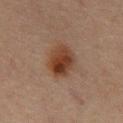• biopsy status — imaged on a skin check; not biopsied
• patient — male, about 70 years old
• tile lighting — cross-polarized illumination
• lesion size — ~4.5 mm (longest diameter)
• body site — the mid back
• acquisition — 15 mm crop, total-body photography